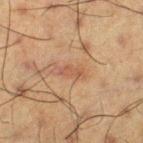Impression: Captured during whole-body skin photography for melanoma surveillance; the lesion was not biopsied.